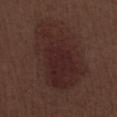Q: Was this lesion biopsied?
A: total-body-photography surveillance lesion; no biopsy
Q: What are the patient's age and sex?
A: male, aged around 70
Q: Where on the body is the lesion?
A: the right lower leg
Q: What is the imaging modality?
A: 15 mm crop, total-body photography
Q: What is the lesion's diameter?
A: ≈9.5 mm
Q: Automated lesion metrics?
A: a shape eccentricity near 0.8 and a symmetry-axis asymmetry near 0.25; a lesion color around L≈25 a*≈18 b*≈18 in CIELAB and a normalized border contrast of about 7.5; a border-irregularity index near 4/10, internal color variation of about 3.5 on a 0–10 scale, and peripheral color asymmetry of about 1; a classifier nevus-likeness of about 80/100 and a detector confidence of about 100 out of 100 that the crop contains a lesion
Q: How was the tile lit?
A: white-light